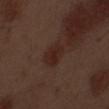A close-up tile cropped from a whole-body skin photograph, about 15 mm across. On the front of the torso. A male subject, aged around 70.